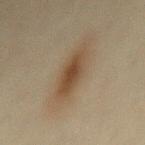workup = no biopsy performed (imaged during a skin exam)
imaging modality = ~15 mm crop, total-body skin-cancer survey
size = ~6.5 mm (longest diameter)
subject = female, aged around 45
body site = the mid back
lighting = cross-polarized
automated metrics = a footprint of about 13 mm² and an eccentricity of roughly 0.95; a lesion color around L≈41 a*≈13 b*≈27 in CIELAB, roughly 9 lightness units darker than nearby skin, and a normalized border contrast of about 8; border irregularity of about 2.5 on a 0–10 scale, internal color variation of about 4 on a 0–10 scale, and peripheral color asymmetry of about 1